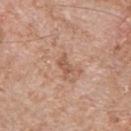biopsy_status: not biopsied; imaged during a skin examination
site: chest
lesion_size:
  long_diameter_mm_approx: 3.0
image:
  source: total-body photography crop
  field_of_view_mm: 15
lighting: white-light
patient:
  sex: male
  age_approx: 55